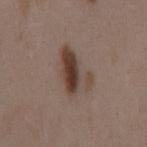| key | value |
|---|---|
| biopsy status | catalogued during a skin exam; not biopsied |
| tile lighting | white-light |
| patient | female, roughly 35 years of age |
| location | the back |
| image source | ~15 mm tile from a whole-body skin photo |